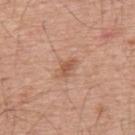* follow-up · imaged on a skin check; not biopsied
* site · the upper back
* patient · male, aged 53 to 57
* image-analysis metrics · a lesion area of about 3 mm², a shape eccentricity near 0.8, and a symmetry-axis asymmetry near 0.45; an average lesion color of about L≈55 a*≈23 b*≈32 (CIELAB), roughly 9 lightness units darker than nearby skin, and a lesion-to-skin contrast of about 6.5 (normalized; higher = more distinct); border irregularity of about 4.5 on a 0–10 scale, a color-variation rating of about 1/10, and radial color variation of about 0.5
* lighting · white-light
* imaging modality · total-body-photography crop, ~15 mm field of view
* diameter · ~2.5 mm (longest diameter)